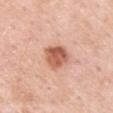workup: total-body-photography surveillance lesion; no biopsy
acquisition: ~15 mm tile from a whole-body skin photo
location: the arm
subject: male, aged 53–57